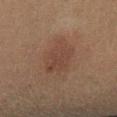workup: imaged on a skin check; not biopsied | lesion diameter: ≈4.5 mm | subject: female, aged around 60 | image source: 15 mm crop, total-body photography | site: the left lower leg | TBP lesion metrics: an area of roughly 11 mm² and an outline eccentricity of about 0.7 (0 = round, 1 = elongated) | illumination: cross-polarized illumination.